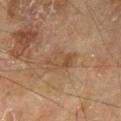Image and clinical context:
Measured at roughly 4 mm in maximum diameter. A 15 mm close-up tile from a total-body photography series done for melanoma screening. A male subject aged approximately 70. Located on the left thigh. The tile uses cross-polarized illumination.